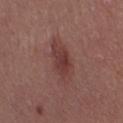The lesion was tiled from a total-body skin photograph and was not biopsied. A 15 mm crop from a total-body photograph taken for skin-cancer surveillance. The patient is a female in their 40s. The lesion is on the left thigh.